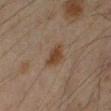Assessment: Captured during whole-body skin photography for melanoma surveillance; the lesion was not biopsied. Background: A male subject aged 43 to 47. Approximately 3.5 mm at its widest. A roughly 15 mm field-of-view crop from a total-body skin photograph. On the left lower leg. Imaged with cross-polarized lighting.